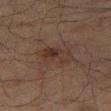Recorded during total-body skin imaging; not selected for excision or biopsy. A roughly 15 mm field-of-view crop from a total-body skin photograph. Imaged with cross-polarized lighting. The total-body-photography lesion software estimated a footprint of about 9 mm², a shape eccentricity near 0.8, and a symmetry-axis asymmetry near 0.2. It also reported a mean CIELAB color near L≈27 a*≈12 b*≈20, roughly 5 lightness units darker than nearby skin, and a normalized lesion–skin contrast near 6. And it measured a border-irregularity index near 2.5/10 and radial color variation of about 2. A male subject, aged 68 to 72. The lesion is located on the right thigh. Longest diameter approximately 4.5 mm.